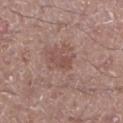Imaged during a routine full-body skin examination; the lesion was not biopsied and no histopathology is available. The total-body-photography lesion software estimated a lesion color around L≈49 a*≈20 b*≈23 in CIELAB, roughly 7 lightness units darker than nearby skin, and a normalized border contrast of about 5.5. The software also gave a border-irregularity rating of about 3/10, internal color variation of about 2 on a 0–10 scale, and peripheral color asymmetry of about 0.5. The lesion is located on the left lower leg. A male patient, in their mid- to late 50s. About 3 mm across. A 15 mm close-up extracted from a 3D total-body photography capture. Captured under white-light illumination.